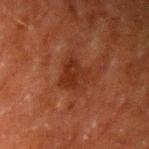The tile uses cross-polarized illumination. A male subject, aged approximately 60. A close-up tile cropped from a whole-body skin photograph, about 15 mm across. From the left upper arm. Approximately 3.5 mm at its widest.